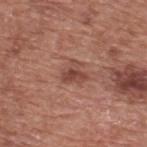Part of a total-body skin-imaging series; this lesion was reviewed on a skin check and was not flagged for biopsy. A region of skin cropped from a whole-body photographic capture, roughly 15 mm wide. Captured under white-light illumination. The lesion's longest dimension is about 3 mm. A male patient aged 73 to 77. From the upper back.